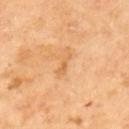This is a cross-polarized tile. About 3 mm across. A male patient, about 70 years old. On the upper back. Cropped from a total-body skin-imaging series; the visible field is about 15 mm. Automated tile analysis of the lesion measured a border-irregularity rating of about 5.5/10, a color-variation rating of about 0/10, and a peripheral color-asymmetry measure near 0.